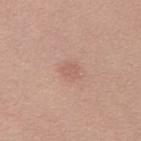<tbp_lesion>
<biopsy_status>not biopsied; imaged during a skin examination</biopsy_status>
<lesion_size>
  <long_diameter_mm_approx>2.0</long_diameter_mm_approx>
</lesion_size>
<site>left thigh</site>
<image>
  <source>total-body photography crop</source>
  <field_of_view_mm>15</field_of_view_mm>
</image>
<lighting>white-light</lighting>
<automated_metrics>
  <area_mm2_approx>3.0</area_mm2_approx>
  <cielab_L>59</cielab_L>
  <cielab_a>22</cielab_a>
  <cielab_b>26</cielab_b>
  <vs_skin_darker_L>7.0</vs_skin_darker_L>
  <vs_skin_contrast_norm>4.5</vs_skin_contrast_norm>
  <border_irregularity_0_10>1.5</border_irregularity_0_10>
  <nevus_likeness_0_100>0</nevus_likeness_0_100>
  <lesion_detection_confidence_0_100>100</lesion_detection_confidence_0_100>
</automated_metrics>
<patient>
  <sex>female</sex>
  <age_approx>45</age_approx>
</patient>
</tbp_lesion>